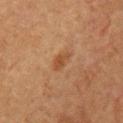  biopsy_status: not biopsied; imaged during a skin examination
  automated_metrics:
    area_mm2_approx: 3.5
    eccentricity: 0.85
    shape_asymmetry: 0.25
    nevus_likeness_0_100: 25
  patient:
    sex: male
    age_approx: 65
  image:
    source: total-body photography crop
    field_of_view_mm: 15
  lesion_size:
    long_diameter_mm_approx: 3.0
  lighting: cross-polarized
  site: chest